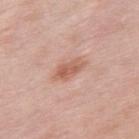The lesion was tiled from a total-body skin photograph and was not biopsied. A male patient, in their mid-50s. A close-up tile cropped from a whole-body skin photograph, about 15 mm across. Longest diameter approximately 4 mm. Automated tile analysis of the lesion measured a lesion area of about 5.5 mm², a shape eccentricity near 0.9, and a symmetry-axis asymmetry near 0.25. The analysis additionally found an average lesion color of about L≈59 a*≈23 b*≈29 (CIELAB), roughly 10 lightness units darker than nearby skin, and a normalized lesion–skin contrast near 7. Imaged with white-light lighting. The lesion is on the mid back.